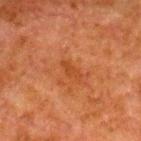  patient:
    sex: male
    age_approx: 80
  automated_metrics:
    area_mm2_approx: 2.5
    cielab_L: 37
    cielab_a: 25
    cielab_b: 35
    vs_skin_darker_L: 6.0
    vs_skin_contrast_norm: 5.5
    border_irregularity_0_10: 3.5
    color_variation_0_10: 0.0
    peripheral_color_asymmetry: 0.0
  lighting: cross-polarized
  site: right lower leg
  image:
    source: total-body photography crop
    field_of_view_mm: 15